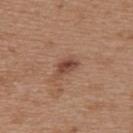The lesion was photographed on a routine skin check and not biopsied; there is no pathology result. A 15 mm crop from a total-body photograph taken for skin-cancer surveillance. About 3 mm across. On the upper back. The subject is a female roughly 40 years of age.